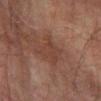Q: Was a biopsy performed?
A: imaged on a skin check; not biopsied
Q: What are the patient's age and sex?
A: male, about 75 years old
Q: Automated lesion metrics?
A: a border-irregularity index near 4.5/10, a within-lesion color-variation index near 1.5/10, and peripheral color asymmetry of about 0.5; a nevus-likeness score of about 0/100 and lesion-presence confidence of about 100/100
Q: What is the imaging modality?
A: ~15 mm tile from a whole-body skin photo
Q: What lighting was used for the tile?
A: cross-polarized
Q: Lesion location?
A: the leg